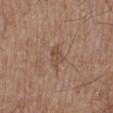<tbp_lesion>
<biopsy_status>not biopsied; imaged during a skin examination</biopsy_status>
<automated_metrics>
  <cielab_L>49</cielab_L>
  <cielab_a>18</cielab_a>
  <cielab_b>28</cielab_b>
  <vs_skin_darker_L>7.0</vs_skin_darker_L>
  <nevus_likeness_0_100>0</nevus_likeness_0_100>
  <lesion_detection_confidence_0_100>100</lesion_detection_confidence_0_100>
</automated_metrics>
<image>
  <source>total-body photography crop</source>
  <field_of_view_mm>15</field_of_view_mm>
</image>
<site>leg</site>
<patient>
  <sex>male</sex>
  <age_approx>65</age_approx>
</patient>
</tbp_lesion>